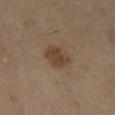| key | value |
|---|---|
| follow-up | imaged on a skin check; not biopsied |
| diameter | about 3 mm |
| image-analysis metrics | a lesion area of about 7.5 mm², a shape eccentricity near 0.55, and two-axis asymmetry of about 0.15; an average lesion color of about L≈39 a*≈16 b*≈26 (CIELAB), about 8 CIELAB-L* units darker than the surrounding skin, and a normalized lesion–skin contrast near 7.5; a color-variation rating of about 2/10 and peripheral color asymmetry of about 0.5; a lesion-detection confidence of about 100/100 |
| site | the left lower leg |
| acquisition | total-body-photography crop, ~15 mm field of view |
| patient | female, aged approximately 50 |
| tile lighting | cross-polarized |